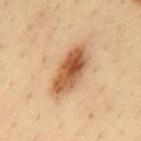Assessment:
Recorded during total-body skin imaging; not selected for excision or biopsy.
Clinical summary:
The recorded lesion diameter is about 6.5 mm. On the mid back. Captured under cross-polarized illumination. A male patient aged 33 to 37. The lesion-visualizer software estimated an area of roughly 15 mm² and a shape eccentricity near 0.9. The software also gave about 14 CIELAB-L* units darker than the surrounding skin. It also reported a border-irregularity rating of about 2.5/10, internal color variation of about 7.5 on a 0–10 scale, and radial color variation of about 2.5. The analysis additionally found a nevus-likeness score of about 90/100. This image is a 15 mm lesion crop taken from a total-body photograph.